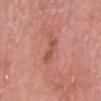This lesion was catalogued during total-body skin photography and was not selected for biopsy.
The lesion-visualizer software estimated a footprint of about 3.5 mm² and a shape-asymmetry score of about 0.4 (0 = symmetric). The analysis additionally found an average lesion color of about L≈53 a*≈27 b*≈29 (CIELAB) and about 8 CIELAB-L* units darker than the surrounding skin. The software also gave a border-irregularity rating of about 4/10, internal color variation of about 0 on a 0–10 scale, and a peripheral color-asymmetry measure near 0. The software also gave lesion-presence confidence of about 100/100.
A male subject aged approximately 85 or older.
A lesion tile, about 15 mm wide, cut from a 3D total-body photograph.
The lesion is located on the head or neck.
The lesion's longest dimension is about 3 mm.